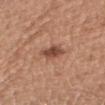Acquisition and patient details: A female patient approximately 40 years of age. Located on the arm. This image is a 15 mm lesion crop taken from a total-body photograph. Imaged with white-light lighting. Longest diameter approximately 3 mm.